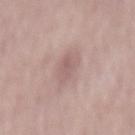Findings:
* follow-up · imaged on a skin check; not biopsied
* subject · male, aged around 60
* location · the mid back
* illumination · white-light
* image · ~15 mm crop, total-body skin-cancer survey